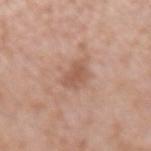The lesion was tiled from a total-body skin photograph and was not biopsied.
The tile uses white-light illumination.
A male subject, aged approximately 55.
Cropped from a whole-body photographic skin survey; the tile spans about 15 mm.
The lesion is located on the left forearm.
Longest diameter approximately 3.5 mm.